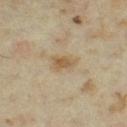Assessment:
Recorded during total-body skin imaging; not selected for excision or biopsy.
Background:
From the left thigh. A female subject about 35 years old. Longest diameter approximately 2.5 mm. The tile uses cross-polarized illumination. Cropped from a total-body skin-imaging series; the visible field is about 15 mm. An algorithmic analysis of the crop reported a lesion-to-skin contrast of about 7.5 (normalized; higher = more distinct).